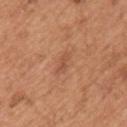{
  "biopsy_status": "not biopsied; imaged during a skin examination",
  "automated_metrics": {
    "cielab_L": 52,
    "cielab_a": 24,
    "cielab_b": 34,
    "vs_skin_darker_L": 7.0,
    "border_irregularity_0_10": 3.0,
    "color_variation_0_10": 2.0,
    "peripheral_color_asymmetry": 0.5
  },
  "patient": {
    "sex": "male",
    "age_approx": 65
  },
  "lighting": "white-light",
  "site": "arm",
  "image": {
    "source": "total-body photography crop",
    "field_of_view_mm": 15
  }
}